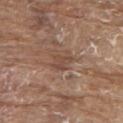| feature | finding |
|---|---|
| patient | male, aged approximately 80 |
| tile lighting | white-light |
| site | the upper back |
| imaging modality | ~15 mm tile from a whole-body skin photo |